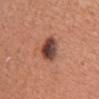biopsy status — no biopsy performed (imaged during a skin exam)
subject — female, in their mid-50s
image — total-body-photography crop, ~15 mm field of view
body site — the chest